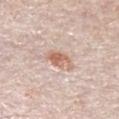Recorded during total-body skin imaging; not selected for excision or biopsy.
A 15 mm close-up tile from a total-body photography series done for melanoma screening.
From the arm.
The total-body-photography lesion software estimated a border-irregularity index near 3.5/10 and peripheral color asymmetry of about 1. And it measured an automated nevus-likeness rating near 95 out of 100 and a detector confidence of about 100 out of 100 that the crop contains a lesion.
The patient is a female roughly 65 years of age.